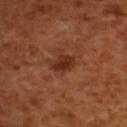Q: Was this lesion biopsied?
A: catalogued during a skin exam; not biopsied
Q: Illumination type?
A: cross-polarized illumination
Q: Lesion location?
A: the upper back
Q: Who is the patient?
A: female, approximately 55 years of age
Q: How large is the lesion?
A: ≈3 mm
Q: What kind of image is this?
A: ~15 mm tile from a whole-body skin photo